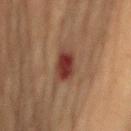Impression: Imaged during a routine full-body skin examination; the lesion was not biopsied and no histopathology is available. Clinical summary: Measured at roughly 4 mm in maximum diameter. A 15 mm close-up tile from a total-body photography series done for melanoma screening. Located on the left upper arm. A female subject in their 80s. Imaged with cross-polarized lighting.